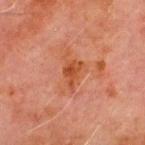Assessment: No biopsy was performed on this lesion — it was imaged during a full skin examination and was not determined to be concerning. Clinical summary: Captured under cross-polarized illumination. From the chest. A close-up tile cropped from a whole-body skin photograph, about 15 mm across. The total-body-photography lesion software estimated a mean CIELAB color near L≈41 a*≈26 b*≈33, roughly 8 lightness units darker than nearby skin, and a lesion-to-skin contrast of about 8 (normalized; higher = more distinct). The software also gave an automated nevus-likeness rating near 0 out of 100 and lesion-presence confidence of about 100/100. A male patient aged approximately 70.